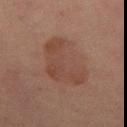The lesion was tiled from a total-body skin photograph and was not biopsied. A 15 mm close-up tile from a total-body photography series done for melanoma screening. The lesion is on the abdomen. A male subject aged 63 to 67.